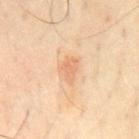This lesion was catalogued during total-body skin photography and was not selected for biopsy.
A male subject, aged approximately 65.
The lesion is on the chest.
A lesion tile, about 15 mm wide, cut from a 3D total-body photograph.